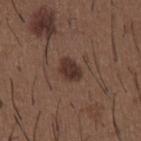biopsy_status: not biopsied; imaged during a skin examination